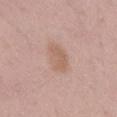Findings:
* patient: male, aged around 55
* image source: ~15 mm tile from a whole-body skin photo
* lighting: white-light
* anatomic site: the back
* lesion size: ~3.5 mm (longest diameter)
* automated lesion analysis: a lesion area of about 7.5 mm², an eccentricity of roughly 0.8, and two-axis asymmetry of about 0.2; a mean CIELAB color near L≈60 a*≈19 b*≈28 and roughly 7 lightness units darker than nearby skin